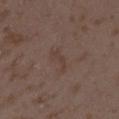• biopsy status · no biopsy performed (imaged during a skin exam)
• lighting · white-light illumination
• anatomic site · the left forearm
• acquisition · ~15 mm crop, total-body skin-cancer survey
• subject · female, in their mid- to late 30s
• lesion size · ~3.5 mm (longest diameter)